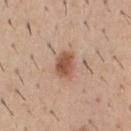Impression: The lesion was photographed on a routine skin check and not biopsied; there is no pathology result. Clinical summary: The lesion is on the chest. Captured under white-light illumination. The recorded lesion diameter is about 3 mm. A male subject, roughly 40 years of age. A roughly 15 mm field-of-view crop from a total-body skin photograph.